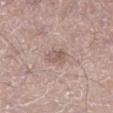biopsy_status: not biopsied; imaged during a skin examination
site: left lower leg
patient:
  sex: male
  age_approx: 55
automated_metrics:
  eccentricity: 0.7
  shape_asymmetry: 0.3
  cielab_L: 56
  cielab_a: 17
  cielab_b: 22
  vs_skin_darker_L: 8.0
  vs_skin_contrast_norm: 5.5
  nevus_likeness_0_100: 0
image:
  source: total-body photography crop
  field_of_view_mm: 15